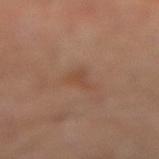The lesion was tiled from a total-body skin photograph and was not biopsied.
A male subject, aged 48 to 52.
Cropped from a total-body skin-imaging series; the visible field is about 15 mm.
From the left leg.